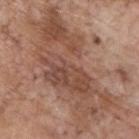The lesion is located on the upper back.
A 15 mm close-up tile from a total-body photography series done for melanoma screening.
An algorithmic analysis of the crop reported a footprint of about 60 mm² and a symmetry-axis asymmetry near 0.35. The software also gave a classifier nevus-likeness of about 0/100 and a lesion-detection confidence of about 50/100.
The lesion's longest dimension is about 14 mm.
A male patient aged 68–72.